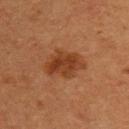workup=no biopsy performed (imaged during a skin exam)
patient=female, aged around 40
site=the upper back
image=~15 mm tile from a whole-body skin photo